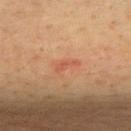The lesion was tiled from a total-body skin photograph and was not biopsied.
The lesion is on the upper back.
A roughly 15 mm field-of-view crop from a total-body skin photograph.
The subject is a male about 40 years old.